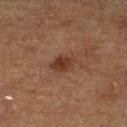biopsy status: imaged on a skin check; not biopsied
lesion diameter: ~3.5 mm (longest diameter)
body site: the left lower leg
automated lesion analysis: a lesion area of about 5 mm², a shape eccentricity near 0.8, and two-axis asymmetry of about 0.2; a lesion color around L≈28 a*≈17 b*≈24 in CIELAB, roughly 8 lightness units darker than nearby skin, and a lesion-to-skin contrast of about 8 (normalized; higher = more distinct)
illumination: cross-polarized illumination
subject: male, aged approximately 75
image source: total-body-photography crop, ~15 mm field of view